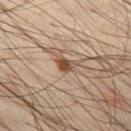Q: Illumination type?
A: cross-polarized illumination
Q: What did automated image analysis measure?
A: a classifier nevus-likeness of about 100/100
Q: Lesion location?
A: the left thigh
Q: What is the lesion's diameter?
A: ~2 mm (longest diameter)
Q: What kind of image is this?
A: ~15 mm tile from a whole-body skin photo
Q: Patient demographics?
A: male, about 50 years old